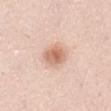workup = catalogued during a skin exam; not biopsied
subject = female, aged around 30
image source = total-body-photography crop, ~15 mm field of view
body site = the front of the torso